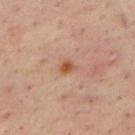notes = catalogued during a skin exam; not biopsied